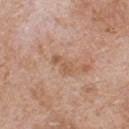The lesion was tiled from a total-body skin photograph and was not biopsied. A 15 mm close-up tile from a total-body photography series done for melanoma screening. The patient is a male aged approximately 65. Measured at roughly 3 mm in maximum diameter. The tile uses white-light illumination. The lesion-visualizer software estimated a mean CIELAB color near L≈57 a*≈20 b*≈32, roughly 8 lightness units darker than nearby skin, and a normalized lesion–skin contrast near 6. From the upper back.